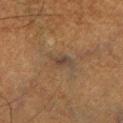image:
  source: total-body photography crop
  field_of_view_mm: 15
patient:
  sex: male
  age_approx: 40
lesion_size:
  long_diameter_mm_approx: 3.0
site: left lower leg
lighting: cross-polarized